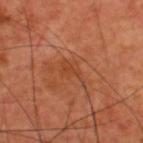The lesion was photographed on a routine skin check and not biopsied; there is no pathology result. A male subject aged 58–62. A region of skin cropped from a whole-body photographic capture, roughly 15 mm wide. The lesion is on the back.